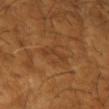biopsy status: no biopsy performed (imaged during a skin exam) | body site: the left forearm | lighting: cross-polarized | size: ~3 mm (longest diameter) | subject: male, aged around 65 | image source: total-body-photography crop, ~15 mm field of view.